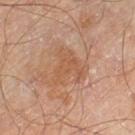No biopsy was performed on this lesion — it was imaged during a full skin examination and was not determined to be concerning.
Automated image analysis of the tile measured a lesion color around L≈53 a*≈21 b*≈32 in CIELAB and roughly 6 lightness units darker than nearby skin. It also reported an automated nevus-likeness rating near 0 out of 100.
Captured under cross-polarized illumination.
A male subject roughly 70 years of age.
A close-up tile cropped from a whole-body skin photograph, about 15 mm across.
From the left thigh.